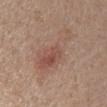Q: Was this lesion biopsied?
A: catalogued during a skin exam; not biopsied
Q: How was this image acquired?
A: total-body-photography crop, ~15 mm field of view
Q: How was the tile lit?
A: white-light
Q: What is the lesion's diameter?
A: ≈8 mm
Q: Lesion location?
A: the arm
Q: Who is the patient?
A: male, roughly 55 years of age